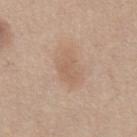The lesion was tiled from a total-body skin photograph and was not biopsied. A roughly 15 mm field-of-view crop from a total-body skin photograph. Automated tile analysis of the lesion measured a lesion area of about 6.5 mm². The analysis additionally found a lesion–skin lightness drop of about 6 and a normalized lesion–skin contrast near 4.5. The analysis additionally found a border-irregularity index near 3.5/10 and internal color variation of about 1.5 on a 0–10 scale. It also reported a classifier nevus-likeness of about 0/100 and a lesion-detection confidence of about 100/100. Imaged with white-light lighting. The lesion's longest dimension is about 3.5 mm. A male subject aged around 60. Located on the abdomen.